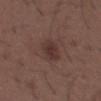The lesion was photographed on a routine skin check and not biopsied; there is no pathology result. From the mid back. A male patient roughly 50 years of age. A 15 mm crop from a total-body photograph taken for skin-cancer surveillance. This is a white-light tile.